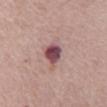Captured during whole-body skin photography for melanoma surveillance; the lesion was not biopsied.
A 15 mm crop from a total-body photograph taken for skin-cancer surveillance.
The lesion is located on the abdomen.
The subject is a female in their mid-60s.